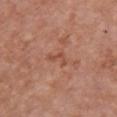Clinical impression:
Imaged during a routine full-body skin examination; the lesion was not biopsied and no histopathology is available.
Context:
The total-body-photography lesion software estimated an eccentricity of roughly 0.85 and a symmetry-axis asymmetry near 0.65. It also reported a lesion color around L≈50 a*≈25 b*≈31 in CIELAB, roughly 7 lightness units darker than nearby skin, and a lesion-to-skin contrast of about 5.5 (normalized; higher = more distinct). A female patient aged 58 to 62. Located on the chest. Approximately 3 mm at its widest. Cropped from a total-body skin-imaging series; the visible field is about 15 mm. This is a white-light tile.